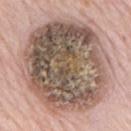Clinical impression: Recorded during total-body skin imaging; not selected for excision or biopsy. Acquisition and patient details: Imaged with white-light lighting. Located on the back. The recorded lesion diameter is about 11 mm. The subject is a male aged 78 to 82. A lesion tile, about 15 mm wide, cut from a 3D total-body photograph.